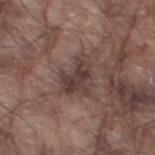The lesion was tiled from a total-body skin photograph and was not biopsied. About 4 mm across. Imaged with white-light lighting. On the left thigh. A roughly 15 mm field-of-view crop from a total-body skin photograph. A male subject, about 80 years old.